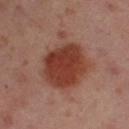biopsy_status: not biopsied; imaged during a skin examination
site: right upper arm
image:
  source: total-body photography crop
  field_of_view_mm: 15
patient:
  sex: female
  age_approx: 40
lighting: cross-polarized
lesion_size:
  long_diameter_mm_approx: 7.0
automated_metrics:
  nevus_likeness_0_100: 100
  lesion_detection_confidence_0_100: 100